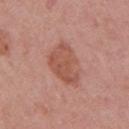biopsy_status: not biopsied; imaged during a skin examination
site: right upper arm
patient:
  sex: female
  age_approx: 55
image:
  source: total-body photography crop
  field_of_view_mm: 15